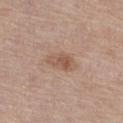Part of a total-body skin-imaging series; this lesion was reviewed on a skin check and was not flagged for biopsy. The lesion's longest dimension is about 4 mm. Located on the left leg. A female subject in their mid- to late 60s. The lesion-visualizer software estimated an outline eccentricity of about 0.85 (0 = round, 1 = elongated) and a shape-asymmetry score of about 0.25 (0 = symmetric). The software also gave a mean CIELAB color near L≈55 a*≈19 b*≈28, a lesion–skin lightness drop of about 9, and a normalized lesion–skin contrast near 6.5. This image is a 15 mm lesion crop taken from a total-body photograph.